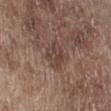Captured during whole-body skin photography for melanoma surveillance; the lesion was not biopsied. Located on the right lower leg. Automated tile analysis of the lesion measured a footprint of about 8 mm², an eccentricity of roughly 0.35, and two-axis asymmetry of about 0.25. The software also gave a border-irregularity index near 3/10 and internal color variation of about 4 on a 0–10 scale. A 15 mm close-up tile from a total-body photography series done for melanoma screening. The subject is a male aged around 70. Captured under white-light illumination. Approximately 3 mm at its widest.